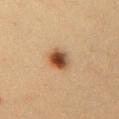image source: ~15 mm tile from a whole-body skin photo
image-analysis metrics: a lesion area of about 6 mm², an outline eccentricity of about 0.5 (0 = round, 1 = elongated), and two-axis asymmetry of about 0.15; a lesion color around L≈42 a*≈19 b*≈31 in CIELAB, a lesion–skin lightness drop of about 15, and a normalized border contrast of about 11.5
patient: female, aged 38–42
illumination: cross-polarized illumination
diameter: ≈3 mm
site: the chest